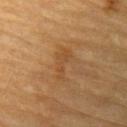Impression:
Imaged during a routine full-body skin examination; the lesion was not biopsied and no histopathology is available.
Context:
The patient is a male roughly 85 years of age or older. The lesion is located on the left upper arm. Cropped from a total-body skin-imaging series; the visible field is about 15 mm.